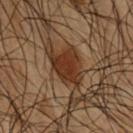This lesion was catalogued during total-body skin photography and was not selected for biopsy. A male subject about 50 years old. Captured under cross-polarized illumination. A roughly 15 mm field-of-view crop from a total-body skin photograph. From the right upper arm. The recorded lesion diameter is about 4.5 mm.